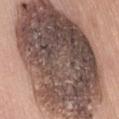• biopsy status · total-body-photography surveillance lesion; no biopsy
• size · about 17 mm
• tile lighting · white-light illumination
• TBP lesion metrics · an area of roughly 130 mm² and two-axis asymmetry of about 0.15; border irregularity of about 2 on a 0–10 scale and internal color variation of about 9.5 on a 0–10 scale; a classifier nevus-likeness of about 0/100 and a lesion-detection confidence of about 85/100
• body site · the abdomen
• subject · female, about 60 years old
• image source · ~15 mm crop, total-body skin-cancer survey